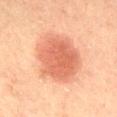- workup — catalogued during a skin exam; not biopsied
- location — the mid back
- TBP lesion metrics — a mean CIELAB color near L≈54 a*≈25 b*≈31, a lesion–skin lightness drop of about 11, and a lesion-to-skin contrast of about 7.5 (normalized; higher = more distinct); an automated nevus-likeness rating near 100 out of 100 and a lesion-detection confidence of about 100/100
- illumination — cross-polarized
- subject — male, aged 58 to 62
- acquisition — ~15 mm crop, total-body skin-cancer survey
- lesion diameter — about 6.5 mm